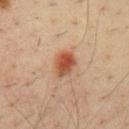Q: Was a biopsy performed?
A: total-body-photography surveillance lesion; no biopsy
Q: What is the anatomic site?
A: the chest
Q: What are the patient's age and sex?
A: male, roughly 35 years of age
Q: What kind of image is this?
A: 15 mm crop, total-body photography